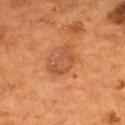follow-up: imaged on a skin check; not biopsied
site: the upper back
lesion diameter: about 4 mm
subject: male, aged 53 to 57
image-analysis metrics: an area of roughly 7.5 mm², an outline eccentricity of about 0.75 (0 = round, 1 = elongated), and a symmetry-axis asymmetry near 0.35; a lesion color around L≈48 a*≈26 b*≈35 in CIELAB, roughly 7 lightness units darker than nearby skin, and a lesion-to-skin contrast of about 5.5 (normalized; higher = more distinct); internal color variation of about 2.5 on a 0–10 scale and radial color variation of about 0.5; an automated nevus-likeness rating near 50 out of 100
tile lighting: cross-polarized illumination
imaging modality: ~15 mm tile from a whole-body skin photo